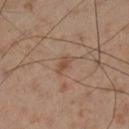biopsy status=total-body-photography surveillance lesion; no biopsy | image=total-body-photography crop, ~15 mm field of view | subject=male, approximately 55 years of age | anatomic site=the left lower leg | size=about 2.5 mm.